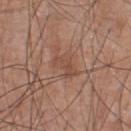Impression: This lesion was catalogued during total-body skin photography and was not selected for biopsy. Context: The lesion is located on the front of the torso. Captured under white-light illumination. A male patient, approximately 55 years of age. Automated tile analysis of the lesion measured a footprint of about 5 mm² and a shape eccentricity near 0.85. The software also gave a border-irregularity rating of about 4.5/10, a color-variation rating of about 2.5/10, and peripheral color asymmetry of about 0.5. It also reported a classifier nevus-likeness of about 0/100. The recorded lesion diameter is about 3.5 mm. A region of skin cropped from a whole-body photographic capture, roughly 15 mm wide.